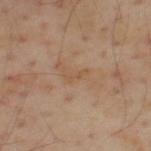Notes:
• patient — male, aged 53–57
• image — total-body-photography crop, ~15 mm field of view
• automated metrics — a lesion area of about 2 mm², a shape eccentricity near 0.95, and two-axis asymmetry of about 0.55; a mean CIELAB color near L≈52 a*≈17 b*≈33; a border-irregularity index near 6/10, a color-variation rating of about 0/10, and a peripheral color-asymmetry measure near 0
• lesion size — about 2.5 mm
• body site — the upper back
• tile lighting — cross-polarized